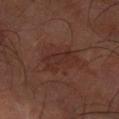| feature | finding |
|---|---|
| follow-up | imaged on a skin check; not biopsied |
| imaging modality | ~15 mm crop, total-body skin-cancer survey |
| anatomic site | the arm |
| subject | male, about 65 years old |